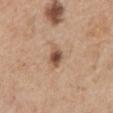A male patient aged 63–67.
Captured under white-light illumination.
A 15 mm crop from a total-body photograph taken for skin-cancer surveillance.
From the mid back.